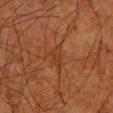The lesion is located on the left lower leg. The subject is a male about 80 years old. Cropped from a total-body skin-imaging series; the visible field is about 15 mm.